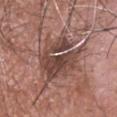Recorded during total-body skin imaging; not selected for excision or biopsy. The total-body-photography lesion software estimated a lesion area of about 21 mm². The analysis additionally found an average lesion color of about L≈44 a*≈19 b*≈23 (CIELAB) and a normalized lesion–skin contrast near 8.5. The software also gave a classifier nevus-likeness of about 10/100. The subject is a male about 65 years old. The lesion's longest dimension is about 6.5 mm. A roughly 15 mm field-of-view crop from a total-body skin photograph. Located on the head or neck.